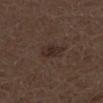notes: imaged on a skin check; not biopsied
location: the left lower leg
imaging modality: total-body-photography crop, ~15 mm field of view
tile lighting: white-light
size: ≈3 mm
patient: male, aged 48–52